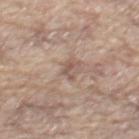Part of a total-body skin-imaging series; this lesion was reviewed on a skin check and was not flagged for biopsy.
Captured under white-light illumination.
A 15 mm close-up extracted from a 3D total-body photography capture.
The subject is a male aged 63 to 67.
Approximately 3 mm at its widest.
On the back.